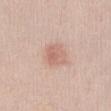Imaged during a routine full-body skin examination; the lesion was not biopsied and no histopathology is available. The lesion is on the abdomen. A female subject about 40 years old. A 15 mm close-up extracted from a 3D total-body photography capture.